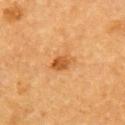- biopsy status: imaged on a skin check; not biopsied
- image-analysis metrics: a lesion color around L≈49 a*≈24 b*≈41 in CIELAB, roughly 10 lightness units darker than nearby skin, and a lesion-to-skin contrast of about 8 (normalized; higher = more distinct); a border-irregularity rating of about 2.5/10, internal color variation of about 3 on a 0–10 scale, and peripheral color asymmetry of about 1
- anatomic site: the right upper arm
- imaging modality: ~15 mm crop, total-body skin-cancer survey
- patient: male, aged 83 or over